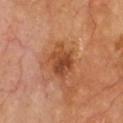– biopsy status — catalogued during a skin exam; not biopsied
– image — ~15 mm tile from a whole-body skin photo
– anatomic site — the chest